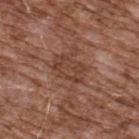<lesion>
  <biopsy_status>not biopsied; imaged during a skin examination</biopsy_status>
  <lighting>white-light</lighting>
  <image>
    <source>total-body photography crop</source>
    <field_of_view_mm>15</field_of_view_mm>
  </image>
  <site>upper back</site>
  <automated_metrics>
    <cielab_L>41</cielab_L>
    <cielab_a>22</cielab_a>
    <cielab_b>27</cielab_b>
    <vs_skin_darker_L>7.0</vs_skin_darker_L>
    <vs_skin_contrast_norm>6.0</vs_skin_contrast_norm>
    <nevus_likeness_0_100>0</nevus_likeness_0_100>
    <lesion_detection_confidence_0_100>100</lesion_detection_confidence_0_100>
  </automated_metrics>
  <patient>
    <sex>male</sex>
    <age_approx>75</age_approx>
  </patient>
</lesion>